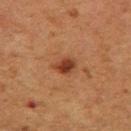Assessment: This lesion was catalogued during total-body skin photography and was not selected for biopsy. Context: The lesion is located on the leg. Measured at roughly 2.5 mm in maximum diameter. A lesion tile, about 15 mm wide, cut from a 3D total-body photograph. The subject is a female in their 50s.